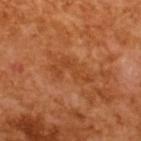Assessment:
The lesion was photographed on a routine skin check and not biopsied; there is no pathology result.
Acquisition and patient details:
A male patient, about 65 years old. The lesion's longest dimension is about 5 mm. Cropped from a total-body skin-imaging series; the visible field is about 15 mm.